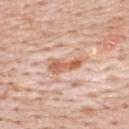biopsy_status: not biopsied; imaged during a skin examination
image:
  source: total-body photography crop
  field_of_view_mm: 15
automated_metrics:
  area_mm2_approx: 7.0
  eccentricity: 0.95
  shape_asymmetry: 0.3
  cielab_L: 63
  cielab_a: 23
  cielab_b: 32
  vs_skin_contrast_norm: 7.5
  border_irregularity_0_10: 4.0
  peripheral_color_asymmetry: 1.0
  nevus_likeness_0_100: 0
  lesion_detection_confidence_0_100: 95
site: upper back
lesion_size:
  long_diameter_mm_approx: 4.5
patient:
  sex: male
  age_approx: 55